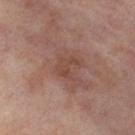Clinical impression: Recorded during total-body skin imaging; not selected for excision or biopsy. Background: Approximately 3 mm at its widest. A 15 mm close-up tile from a total-body photography series done for melanoma screening. Imaged with cross-polarized lighting. Located on the left thigh. A female patient, aged around 55.